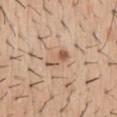Cropped from a total-body skin-imaging series; the visible field is about 15 mm.
The total-body-photography lesion software estimated an area of roughly 4.5 mm², an eccentricity of roughly 0.9, and a shape-asymmetry score of about 0.4 (0 = symmetric). The software also gave an average lesion color of about L≈58 a*≈18 b*≈31 (CIELAB), roughly 10 lightness units darker than nearby skin, and a normalized border contrast of about 7. The software also gave a color-variation rating of about 2/10 and a peripheral color-asymmetry measure near 0.5. It also reported an automated nevus-likeness rating near 20 out of 100 and a lesion-detection confidence of about 100/100.
Located on the abdomen.
A male subject, aged around 40.